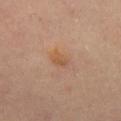Clinical impression: Captured during whole-body skin photography for melanoma surveillance; the lesion was not biopsied. Clinical summary: Cropped from a whole-body photographic skin survey; the tile spans about 15 mm. Located on the mid back. Imaged with cross-polarized lighting. A male patient, roughly 45 years of age.